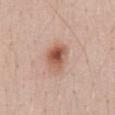About 3.5 mm across. Located on the abdomen. A male patient, aged 53 to 57. This image is a 15 mm lesion crop taken from a total-body photograph. This is a white-light tile.